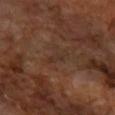Part of a total-body skin-imaging series; this lesion was reviewed on a skin check and was not flagged for biopsy.